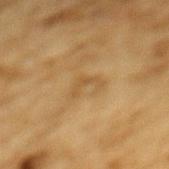Assessment:
Imaged during a routine full-body skin examination; the lesion was not biopsied and no histopathology is available.
Clinical summary:
The subject is a male roughly 85 years of age or older. On the mid back. A lesion tile, about 15 mm wide, cut from a 3D total-body photograph.